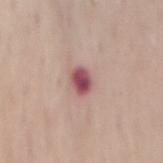The tile uses white-light illumination.
A male patient about 65 years old.
A region of skin cropped from a whole-body photographic capture, roughly 15 mm wide.
The lesion's longest dimension is about 2.5 mm.
Located on the lower back.